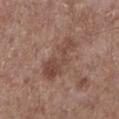{"biopsy_status": "not biopsied; imaged during a skin examination", "patient": {"sex": "male", "age_approx": 60}, "site": "left lower leg", "image": {"source": "total-body photography crop", "field_of_view_mm": 15}, "lighting": "white-light", "automated_metrics": {"color_variation_0_10": 3.5, "peripheral_color_asymmetry": 1.0, "nevus_likeness_0_100": 0, "lesion_detection_confidence_0_100": 100}}